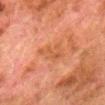Clinical impression:
Part of a total-body skin-imaging series; this lesion was reviewed on a skin check and was not flagged for biopsy.
Clinical summary:
A roughly 15 mm field-of-view crop from a total-body skin photograph. About 4.5 mm across. Located on the leg. A male subject, aged 78–82. Imaged with cross-polarized lighting.